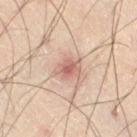The lesion was tiled from a total-body skin photograph and was not biopsied.
A 15 mm close-up tile from a total-body photography series done for melanoma screening.
The subject is a male approximately 50 years of age.
The total-body-photography lesion software estimated a lesion color around L≈55 a*≈19 b*≈24 in CIELAB and a normalized lesion–skin contrast near 7. And it measured an automated nevus-likeness rating near 45 out of 100 and a detector confidence of about 100 out of 100 that the crop contains a lesion.
This is a cross-polarized tile.
About 3 mm across.
The lesion is on the right thigh.